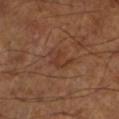No biopsy was performed on this lesion — it was imaged during a full skin examination and was not determined to be concerning.
A lesion tile, about 15 mm wide, cut from a 3D total-body photograph.
The patient is aged around 65.
The tile uses cross-polarized illumination.
Located on the left lower leg.
Measured at roughly 3.5 mm in maximum diameter.
Automated tile analysis of the lesion measured an area of roughly 6 mm², a shape eccentricity near 0.75, and two-axis asymmetry of about 0.45. The analysis additionally found a mean CIELAB color near L≈36 a*≈20 b*≈28 and roughly 6 lightness units darker than nearby skin.